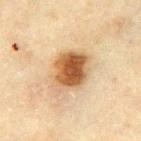Recorded during total-body skin imaging; not selected for excision or biopsy. This is a cross-polarized tile. Cropped from a total-body skin-imaging series; the visible field is about 15 mm. The subject is a male about 70 years old. About 5 mm across. The lesion is on the front of the torso. An algorithmic analysis of the crop reported an area of roughly 15 mm², an eccentricity of roughly 0.55, and a symmetry-axis asymmetry near 0.15. The analysis additionally found an average lesion color of about L≈48 a*≈18 b*≈33 (CIELAB), roughly 14 lightness units darker than nearby skin, and a normalized lesion–skin contrast near 11.